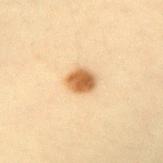Q: Was this lesion biopsied?
A: imaged on a skin check; not biopsied
Q: How was this image acquired?
A: 15 mm crop, total-body photography
Q: Who is the patient?
A: female, aged 28 to 32
Q: How large is the lesion?
A: about 3 mm
Q: Lesion location?
A: the right forearm
Q: Illumination type?
A: cross-polarized illumination